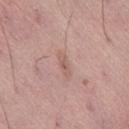image:
  source: total-body photography crop
  field_of_view_mm: 15
site: leg
patient:
  sex: male
  age_approx: 55
lesion_size:
  long_diameter_mm_approx: 3.5
automated_metrics:
  eccentricity: 0.95
  shape_asymmetry: 0.25
  cielab_L: 58
  cielab_a: 20
  cielab_b: 24
  vs_skin_darker_L: 7.0
  vs_skin_contrast_norm: 5.0